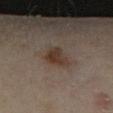Q: Was a biopsy performed?
A: catalogued during a skin exam; not biopsied
Q: Lesion location?
A: the right lower leg
Q: Who is the patient?
A: female, aged approximately 35
Q: Automated lesion metrics?
A: border irregularity of about 2.5 on a 0–10 scale, internal color variation of about 4 on a 0–10 scale, and peripheral color asymmetry of about 1.5; an automated nevus-likeness rating near 95 out of 100 and a detector confidence of about 100 out of 100 that the crop contains a lesion
Q: What is the imaging modality?
A: ~15 mm crop, total-body skin-cancer survey
Q: What is the lesion's diameter?
A: about 3.5 mm